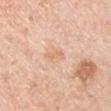biopsy status: total-body-photography surveillance lesion; no biopsy | image source: ~15 mm tile from a whole-body skin photo | body site: the arm | subject: male, approximately 40 years of age.